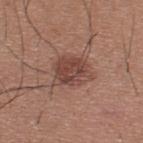Part of a total-body skin-imaging series; this lesion was reviewed on a skin check and was not flagged for biopsy.
The patient is a male roughly 35 years of age.
This image is a 15 mm lesion crop taken from a total-body photograph.
The lesion's longest dimension is about 4 mm.
Captured under white-light illumination.
Located on the upper back.